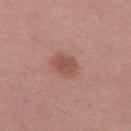follow-up — catalogued during a skin exam; not biopsied
tile lighting — white-light
diameter — ≈3 mm
subject — female, roughly 40 years of age
body site — the left thigh
image — ~15 mm crop, total-body skin-cancer survey